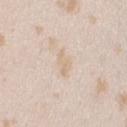biopsy_status: not biopsied; imaged during a skin examination
site: right upper arm
lesion_size:
  long_diameter_mm_approx: 3.0
automated_metrics:
  nevus_likeness_0_100: 0
patient:
  sex: female
  age_approx: 25
lighting: white-light
image:
  source: total-body photography crop
  field_of_view_mm: 15